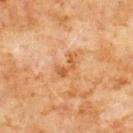Clinical impression:
This lesion was catalogued during total-body skin photography and was not selected for biopsy.
Context:
The lesion is located on the chest. A 15 mm crop from a total-body photograph taken for skin-cancer surveillance. This is a cross-polarized tile. The subject is a male aged 58–62.